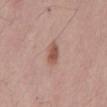Notes:
* biopsy status · imaged on a skin check; not biopsied
* lesion diameter · ~2.5 mm (longest diameter)
* imaging modality · ~15 mm crop, total-body skin-cancer survey
* anatomic site · the back
* TBP lesion metrics · a lesion area of about 4 mm², a shape eccentricity near 0.75, and a symmetry-axis asymmetry near 0.25; border irregularity of about 2 on a 0–10 scale, a color-variation rating of about 2/10, and a peripheral color-asymmetry measure near 0.5; a classifier nevus-likeness of about 90/100
* patient · male, about 55 years old
* tile lighting · white-light